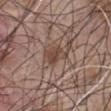Case summary:
- workup — no biopsy performed (imaged during a skin exam)
- size — ≈3.5 mm
- automated metrics — a shape eccentricity near 0.85; a lesion color around L≈44 a*≈17 b*≈25 in CIELAB, about 9 CIELAB-L* units darker than the surrounding skin, and a normalized lesion–skin contrast near 7.5; a border-irregularity index near 4/10, internal color variation of about 2.5 on a 0–10 scale, and a peripheral color-asymmetry measure near 1; a classifier nevus-likeness of about 35/100 and lesion-presence confidence of about 100/100
- lighting — white-light illumination
- patient — male, aged approximately 45
- body site — the chest
- image — 15 mm crop, total-body photography